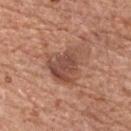Captured during whole-body skin photography for melanoma surveillance; the lesion was not biopsied.
Approximately 4.5 mm at its widest.
A roughly 15 mm field-of-view crop from a total-body skin photograph.
On the chest.
A male patient, in their 70s.
Automated tile analysis of the lesion measured a footprint of about 13 mm², a shape eccentricity near 0.6, and a shape-asymmetry score of about 0.4 (0 = symmetric). And it measured a lesion color around L≈48 a*≈22 b*≈28 in CIELAB, about 10 CIELAB-L* units darker than the surrounding skin, and a lesion-to-skin contrast of about 7.5 (normalized; higher = more distinct). It also reported a classifier nevus-likeness of about 10/100 and lesion-presence confidence of about 100/100.
Captured under white-light illumination.